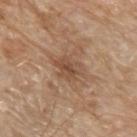{"biopsy_status": "not biopsied; imaged during a skin examination", "lighting": "white-light", "image": {"source": "total-body photography crop", "field_of_view_mm": 15}, "lesion_size": {"long_diameter_mm_approx": 4.0}, "site": "left forearm", "automated_metrics": {"area_mm2_approx": 6.5, "eccentricity": 0.7, "shape_asymmetry": 0.3, "lesion_detection_confidence_0_100": 100}, "patient": {"sex": "male", "age_approx": 80}}